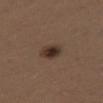Clinical impression:
Imaged during a routine full-body skin examination; the lesion was not biopsied and no histopathology is available.